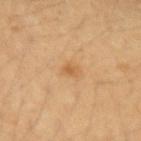The lesion was photographed on a routine skin check and not biopsied; there is no pathology result.
On the left forearm.
A 15 mm close-up extracted from a 3D total-body photography capture.
Automated tile analysis of the lesion measured a lesion color around L≈55 a*≈20 b*≈39 in CIELAB, roughly 8 lightness units darker than nearby skin, and a lesion-to-skin contrast of about 6 (normalized; higher = more distinct). It also reported a border-irregularity rating of about 2.5/10 and a within-lesion color-variation index near 1/10.
This is a cross-polarized tile.
A female subject roughly 30 years of age.